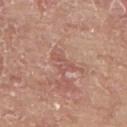workup: imaged on a skin check; not biopsied | automated metrics: a footprint of about 4 mm², a shape eccentricity near 0.9, and a symmetry-axis asymmetry near 0.6; an average lesion color of about L≈55 a*≈24 b*≈26 (CIELAB) | imaging modality: 15 mm crop, total-body photography | subject: male, aged around 65 | anatomic site: the back | tile lighting: white-light illumination | lesion diameter: ≈3.5 mm.